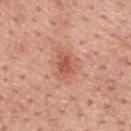No biopsy was performed on this lesion — it was imaged during a full skin examination and was not determined to be concerning.
The subject is a male roughly 60 years of age.
The lesion is on the upper back.
A 15 mm close-up tile from a total-body photography series done for melanoma screening.